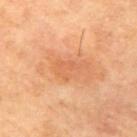Q: Was a biopsy performed?
A: imaged on a skin check; not biopsied
Q: Patient demographics?
A: female, roughly 70 years of age
Q: What is the imaging modality?
A: ~15 mm crop, total-body skin-cancer survey
Q: Lesion location?
A: the right upper arm